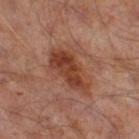{"biopsy_status": "not biopsied; imaged during a skin examination", "lighting": "cross-polarized", "image": {"source": "total-body photography crop", "field_of_view_mm": 15}, "lesion_size": {"long_diameter_mm_approx": 6.5}, "patient": {"sex": "male", "age_approx": 65}, "site": "right thigh"}